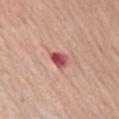No biopsy was performed on this lesion — it was imaged during a full skin examination and was not determined to be concerning. The tile uses white-light illumination. The lesion is located on the right upper arm. This image is a 15 mm lesion crop taken from a total-body photograph. A male subject approximately 65 years of age.